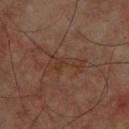Assessment: No biopsy was performed on this lesion — it was imaged during a full skin examination and was not determined to be concerning.